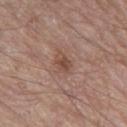biopsy status: no biopsy performed (imaged during a skin exam) | image: 15 mm crop, total-body photography | lighting: white-light illumination | anatomic site: the leg | size: ≈2.5 mm | patient: male, roughly 65 years of age | TBP lesion metrics: an area of roughly 4 mm², an outline eccentricity of about 0.8 (0 = round, 1 = elongated), and a symmetry-axis asymmetry near 0.2; a classifier nevus-likeness of about 0/100 and lesion-presence confidence of about 100/100.